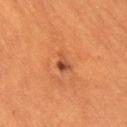– biopsy status — catalogued during a skin exam; not biopsied
– subject — female, roughly 55 years of age
– site — the right thigh
– acquisition — ~15 mm tile from a whole-body skin photo
– size — ~2.5 mm (longest diameter)
– lighting — cross-polarized illumination